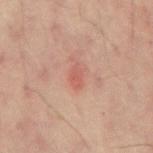Q: Was this lesion biopsied?
A: no biopsy performed (imaged during a skin exam)
Q: What is the anatomic site?
A: the mid back
Q: How was this image acquired?
A: ~15 mm tile from a whole-body skin photo
Q: What are the patient's age and sex?
A: male, approximately 60 years of age